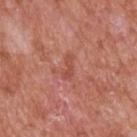Clinical impression: The lesion was tiled from a total-body skin photograph and was not biopsied. Acquisition and patient details: A male subject in their mid- to late 60s. The lesion is on the upper back. Imaged with white-light lighting. Cropped from a whole-body photographic skin survey; the tile spans about 15 mm. Measured at roughly 3 mm in maximum diameter. Automated image analysis of the tile measured peripheral color asymmetry of about 0.